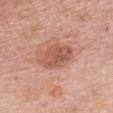biopsy status = total-body-photography surveillance lesion; no biopsy | lighting = white-light illumination | diameter = about 5 mm | subject = female, aged 58 to 62 | image = 15 mm crop, total-body photography | location = the chest.